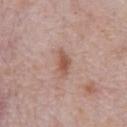Notes:
- subject — male, in their 70s
- anatomic site — the chest
- acquisition — 15 mm crop, total-body photography
- illumination — white-light illumination
- size — ≈3.5 mm